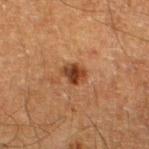{"biopsy_status": "not biopsied; imaged during a skin examination", "site": "leg", "image": {"source": "total-body photography crop", "field_of_view_mm": 15}, "patient": {"sex": "male", "age_approx": 65}, "automated_metrics": {"vs_skin_darker_L": 12.0, "vs_skin_contrast_norm": 10.0, "border_irregularity_0_10": 1.5, "color_variation_0_10": 5.0, "peripheral_color_asymmetry": 1.5}}